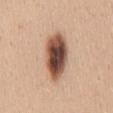Clinical impression: The lesion was tiled from a total-body skin photograph and was not biopsied. Background: The subject is a female in their mid- to late 40s. Captured under white-light illumination. A close-up tile cropped from a whole-body skin photograph, about 15 mm across. Measured at roughly 7 mm in maximum diameter. On the mid back.